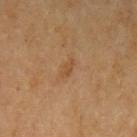  biopsy_status: not biopsied; imaged during a skin examination
  image:
    source: total-body photography crop
    field_of_view_mm: 15
  site: arm
  lesion_size:
    long_diameter_mm_approx: 2.5
  patient:
    sex: female
    age_approx: 70